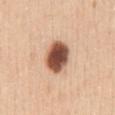  biopsy_status: not biopsied; imaged during a skin examination
  image:
    source: total-body photography crop
    field_of_view_mm: 15
  site: abdomen
  automated_metrics:
    area_mm2_approx: 12.0
    eccentricity: 0.65
    shape_asymmetry: 0.1
    border_irregularity_0_10: 1.0
    peripheral_color_asymmetry: 2.0
    nevus_likeness_0_100: 100
  patient:
    sex: female
    age_approx: 30
  lighting: white-light